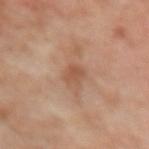biopsy status = catalogued during a skin exam; not biopsied | diameter = ≈2.5 mm | subject = female, aged 48 to 52 | image-analysis metrics = an area of roughly 3.5 mm², an eccentricity of roughly 0.7, and two-axis asymmetry of about 0.25; an automated nevus-likeness rating near 0 out of 100 | anatomic site = the left forearm | tile lighting = cross-polarized | imaging modality = 15 mm crop, total-body photography.